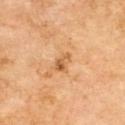Recorded during total-body skin imaging; not selected for excision or biopsy. The tile uses cross-polarized illumination. On the upper back. A male subject aged around 70. A 15 mm crop from a total-body photograph taken for skin-cancer surveillance. Longest diameter approximately 2.5 mm.